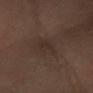{
  "biopsy_status": "not biopsied; imaged during a skin examination",
  "image": {
    "source": "total-body photography crop",
    "field_of_view_mm": 15
  },
  "site": "right forearm",
  "lesion_size": {
    "long_diameter_mm_approx": 3.0
  },
  "lighting": "cross-polarized",
  "patient": {
    "sex": "female"
  },
  "automated_metrics": {
    "area_mm2_approx": 4.5,
    "eccentricity": 0.6,
    "shape_asymmetry": 0.35,
    "cielab_L": 29,
    "cielab_a": 14,
    "cielab_b": 20,
    "vs_skin_darker_L": 4.0,
    "vs_skin_contrast_norm": 4.5,
    "color_variation_0_10": 1.5,
    "peripheral_color_asymmetry": 0.5,
    "nevus_likeness_0_100": 5,
    "lesion_detection_confidence_0_100": 100
  }
}